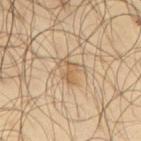notes = no biopsy performed (imaged during a skin exam) | imaging modality = total-body-photography crop, ~15 mm field of view | patient = male, aged 63 to 67 | location = the upper back | lighting = cross-polarized.